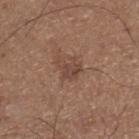About 3 mm across.
Captured under white-light illumination.
The subject is a male aged 73 to 77.
From the right lower leg.
A lesion tile, about 15 mm wide, cut from a 3D total-body photograph.